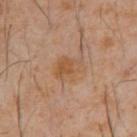Notes:
• workup: total-body-photography surveillance lesion; no biopsy
• anatomic site: the abdomen
• tile lighting: cross-polarized
• patient: male, in their 60s
• imaging modality: 15 mm crop, total-body photography
• diameter: ~3.5 mm (longest diameter)
• image-analysis metrics: a border-irregularity rating of about 3.5/10, a color-variation rating of about 4.5/10, and a peripheral color-asymmetry measure near 1.5